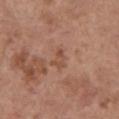Assessment:
Recorded during total-body skin imaging; not selected for excision or biopsy.
Clinical summary:
The lesion is on the left upper arm. Automated tile analysis of the lesion measured a symmetry-axis asymmetry near 0.4. And it measured a border-irregularity rating of about 4/10, a color-variation rating of about 1/10, and radial color variation of about 0. And it measured a nevus-likeness score of about 0/100 and a lesion-detection confidence of about 100/100. Approximately 2.5 mm at its widest. A 15 mm close-up tile from a total-body photography series done for melanoma screening. A female patient aged approximately 65. This is a white-light tile.